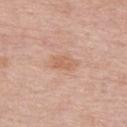Recorded during total-body skin imaging; not selected for excision or biopsy. A male subject in their mid-70s. Imaged with white-light lighting. From the back. Measured at roughly 3 mm in maximum diameter. Cropped from a total-body skin-imaging series; the visible field is about 15 mm.